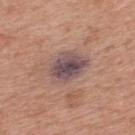No biopsy was performed on this lesion — it was imaged during a full skin examination and was not determined to be concerning. The tile uses white-light illumination. A male subject, in their mid- to late 50s. A 15 mm crop from a total-body photograph taken for skin-cancer surveillance. The lesion's longest dimension is about 5 mm. The lesion is on the back.